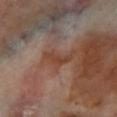{"biopsy_status": "not biopsied; imaged during a skin examination", "site": "left lower leg", "lighting": "cross-polarized", "automated_metrics": {"nevus_likeness_0_100": 0}, "image": {"source": "total-body photography crop", "field_of_view_mm": 15}, "lesion_size": {"long_diameter_mm_approx": 3.5}, "patient": {"sex": "male", "age_approx": 70}}